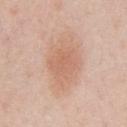Q: Is there a histopathology result?
A: no biopsy performed (imaged during a skin exam)
Q: Where on the body is the lesion?
A: the abdomen
Q: What are the patient's age and sex?
A: male, aged 58 to 62
Q: What kind of image is this?
A: ~15 mm crop, total-body skin-cancer survey